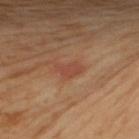The lesion was photographed on a routine skin check and not biopsied; there is no pathology result. The subject is a female roughly 55 years of age. This image is a 15 mm lesion crop taken from a total-body photograph. Imaged with cross-polarized lighting. Approximately 2.5 mm at its widest. From the left upper arm.